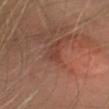The lesion was photographed on a routine skin check and not biopsied; there is no pathology result.
The lesion is located on the head or neck.
The tile uses cross-polarized illumination.
A female subject, aged 33 to 37.
A close-up tile cropped from a whole-body skin photograph, about 15 mm across.
Automated image analysis of the tile measured an area of roughly 1.5 mm². And it measured a peripheral color-asymmetry measure near 0. It also reported a lesion-detection confidence of about 95/100.
The recorded lesion diameter is about 1.5 mm.